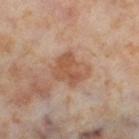{
  "biopsy_status": "not biopsied; imaged during a skin examination",
  "patient": {
    "sex": "female",
    "age_approx": 55
  },
  "lighting": "cross-polarized",
  "image": {
    "source": "total-body photography crop",
    "field_of_view_mm": 15
  },
  "automated_metrics": {
    "border_irregularity_0_10": 2.5,
    "color_variation_0_10": 4.0,
    "peripheral_color_asymmetry": 1.5,
    "nevus_likeness_0_100": 0,
    "lesion_detection_confidence_0_100": 100
  },
  "site": "right thigh"
}